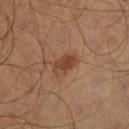biopsy status — total-body-photography surveillance lesion; no biopsy
acquisition — total-body-photography crop, ~15 mm field of view
illumination — cross-polarized illumination
subject — male, roughly 40 years of age
size — ≈3.5 mm
location — the leg